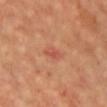| key | value |
|---|---|
| size | ~3 mm (longest diameter) |
| image | 15 mm crop, total-body photography |
| patient | male, aged 63 to 67 |
| site | the chest |
| image-analysis metrics | a footprint of about 3 mm², an eccentricity of roughly 0.85, and two-axis asymmetry of about 0.25; lesion-presence confidence of about 100/100 |